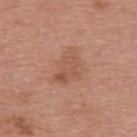Notes:
• site · the upper back
• imaging modality · total-body-photography crop, ~15 mm field of view
• patient · female, roughly 50 years of age